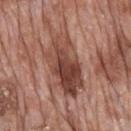Q: Is there a histopathology result?
A: catalogued during a skin exam; not biopsied
Q: What did automated image analysis measure?
A: a mean CIELAB color near L≈45 a*≈23 b*≈26, a lesion–skin lightness drop of about 12, and a normalized lesion–skin contrast near 9
Q: Lesion location?
A: the mid back
Q: How large is the lesion?
A: ~7.5 mm (longest diameter)
Q: How was this image acquired?
A: total-body-photography crop, ~15 mm field of view
Q: Who is the patient?
A: male, aged 68 to 72